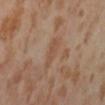The lesion is located on the abdomen. The subject is a female roughly 55 years of age. A 15 mm close-up tile from a total-body photography series done for melanoma screening.